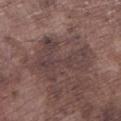{"biopsy_status": "not biopsied; imaged during a skin examination", "image": {"source": "total-body photography crop", "field_of_view_mm": 15}, "site": "left lower leg", "lighting": "white-light", "patient": {"sex": "male", "age_approx": 75}, "lesion_size": {"long_diameter_mm_approx": 6.5}}